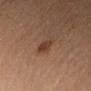body site: the left forearm
patient: female, approximately 40 years of age
lesion diameter: about 2.5 mm
imaging modality: 15 mm crop, total-body photography
lighting: cross-polarized illumination